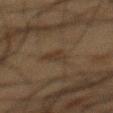| field | value |
|---|---|
| workup | total-body-photography surveillance lesion; no biopsy |
| image-analysis metrics | a lesion area of about 4 mm² and a shape eccentricity near 0.8; a lesion color around L≈26 a*≈10 b*≈21 in CIELAB, roughly 5 lightness units darker than nearby skin, and a lesion-to-skin contrast of about 6 (normalized; higher = more distinct); border irregularity of about 4 on a 0–10 scale |
| patient | male, aged 58–62 |
| location | the mid back |
| tile lighting | cross-polarized |
| imaging modality | ~15 mm tile from a whole-body skin photo |